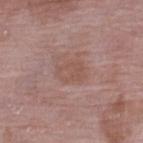biopsy_status: not biopsied; imaged during a skin examination
site: left lower leg
lighting: white-light
lesion_size:
  long_diameter_mm_approx: 3.0
image:
  source: total-body photography crop
  field_of_view_mm: 15
automated_metrics:
  area_mm2_approx: 6.0
  eccentricity: 0.75
patient:
  sex: female
  age_approx: 70